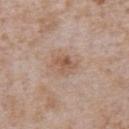Q: Was a biopsy performed?
A: imaged on a skin check; not biopsied
Q: Lesion location?
A: the chest
Q: What are the patient's age and sex?
A: male, aged approximately 65
Q: What is the imaging modality?
A: ~15 mm crop, total-body skin-cancer survey
Q: Illumination type?
A: white-light illumination
Q: How large is the lesion?
A: about 2.5 mm